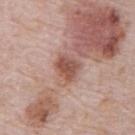Background: A male patient, about 70 years old. A 15 mm crop from a total-body photograph taken for skin-cancer surveillance. The lesion is located on the abdomen.